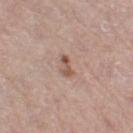Findings:
* biopsy status: imaged on a skin check; not biopsied
* patient: female, aged 63 to 67
* anatomic site: the right thigh
* lighting: white-light illumination
* image source: total-body-photography crop, ~15 mm field of view
* size: ≈2.5 mm